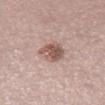Impression: The lesion was tiled from a total-body skin photograph and was not biopsied. Image and clinical context: The lesion is located on the right thigh. A lesion tile, about 15 mm wide, cut from a 3D total-body photograph. A male subject in their 70s.